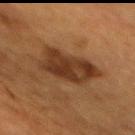{"biopsy_status": "not biopsied; imaged during a skin examination", "site": "mid back", "automated_metrics": {"area_mm2_approx": 18.0, "eccentricity": 0.75, "vs_skin_darker_L": 10.0, "border_irregularity_0_10": 3.0, "color_variation_0_10": 4.0, "peripheral_color_asymmetry": 1.5}, "lighting": "cross-polarized", "lesion_size": {"long_diameter_mm_approx": 6.0}, "image": {"source": "total-body photography crop", "field_of_view_mm": 15}, "patient": {"sex": "female", "age_approx": 55}}